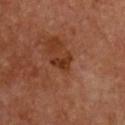notes — imaged on a skin check; not biopsied | image-analysis metrics — a lesion color around L≈33 a*≈25 b*≈33 in CIELAB, about 9 CIELAB-L* units darker than the surrounding skin, and a normalized lesion–skin contrast near 8.5; border irregularity of about 4.5 on a 0–10 scale and peripheral color asymmetry of about 0; a classifier nevus-likeness of about 0/100 and lesion-presence confidence of about 100/100 | imaging modality — ~15 mm tile from a whole-body skin photo | location — the chest | subject — female, roughly 65 years of age | illumination — cross-polarized illumination | size — ~2.5 mm (longest diameter).